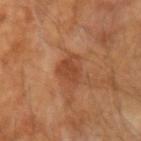Recorded during total-body skin imaging; not selected for excision or biopsy. The subject is a male in their mid-60s. Longest diameter approximately 3 mm. A 15 mm close-up extracted from a 3D total-body photography capture. Captured under cross-polarized illumination. The total-body-photography lesion software estimated roughly 8 lightness units darker than nearby skin and a normalized lesion–skin contrast near 6.5. It also reported border irregularity of about 3 on a 0–10 scale and peripheral color asymmetry of about 1. It also reported a classifier nevus-likeness of about 25/100 and a lesion-detection confidence of about 100/100. On the right upper arm.